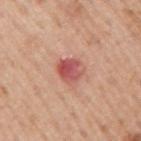Recorded during total-body skin imaging; not selected for excision or biopsy.
A male subject, roughly 75 years of age.
A region of skin cropped from a whole-body photographic capture, roughly 15 mm wide.
Located on the right upper arm.